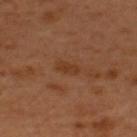<lesion>
<biopsy_status>not biopsied; imaged during a skin examination</biopsy_status>
<site>upper back</site>
<patient>
  <sex>male</sex>
  <age_approx>50</age_approx>
</patient>
<lighting>cross-polarized</lighting>
<lesion_size>
  <long_diameter_mm_approx>2.5</long_diameter_mm_approx>
</lesion_size>
<image>
  <source>total-body photography crop</source>
  <field_of_view_mm>15</field_of_view_mm>
</image>
</lesion>